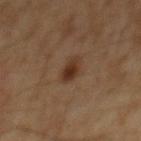follow-up — catalogued during a skin exam; not biopsied | image — ~15 mm tile from a whole-body skin photo | lesion diameter — ~3 mm (longest diameter) | illumination — cross-polarized illumination | site — the back | subject — male, roughly 60 years of age.